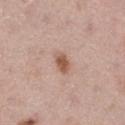The lesion was tiled from a total-body skin photograph and was not biopsied.
A roughly 15 mm field-of-view crop from a total-body skin photograph.
The lesion is on the right thigh.
Automated image analysis of the tile measured an average lesion color of about L≈56 a*≈20 b*≈29 (CIELAB), roughly 12 lightness units darker than nearby skin, and a normalized lesion–skin contrast near 8.5.
A male subject, roughly 75 years of age.
Captured under white-light illumination.